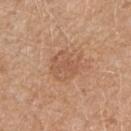Assessment:
This lesion was catalogued during total-body skin photography and was not selected for biopsy.
Acquisition and patient details:
A female subject, roughly 55 years of age. A 15 mm crop from a total-body photograph taken for skin-cancer surveillance. Longest diameter approximately 4 mm. This is a white-light tile. Located on the right forearm.